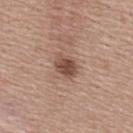This lesion was catalogued during total-body skin photography and was not selected for biopsy.
A female subject in their 60s.
The lesion is located on the upper back.
A 15 mm close-up tile from a total-body photography series done for melanoma screening.